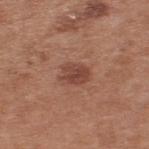A male patient roughly 55 years of age. The lesion is on the back. Cropped from a total-body skin-imaging series; the visible field is about 15 mm. Approximately 3.5 mm at its widest. Automated tile analysis of the lesion measured an outline eccentricity of about 0.65 (0 = round, 1 = elongated) and two-axis asymmetry of about 0.25. And it measured a lesion color around L≈45 a*≈24 b*≈28 in CIELAB and a lesion-to-skin contrast of about 7 (normalized; higher = more distinct). It also reported a border-irregularity rating of about 3/10 and a within-lesion color-variation index near 3/10. The software also gave a lesion-detection confidence of about 100/100. The tile uses white-light illumination.